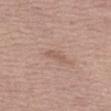This lesion was catalogued during total-body skin photography and was not selected for biopsy.
A 15 mm close-up extracted from a 3D total-body photography capture.
The tile uses white-light illumination.
About 2.5 mm across.
The total-body-photography lesion software estimated a lesion–skin lightness drop of about 7. The software also gave border irregularity of about 3.5 on a 0–10 scale, a color-variation rating of about 0/10, and peripheral color asymmetry of about 0. The software also gave an automated nevus-likeness rating near 0 out of 100 and a detector confidence of about 100 out of 100 that the crop contains a lesion.
A male patient aged 73–77.
Located on the left thigh.